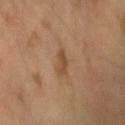follow-up: imaged on a skin check; not biopsied
image: 15 mm crop, total-body photography
patient: male, aged 63 to 67
diameter: ≈3 mm
lighting: cross-polarized illumination
automated lesion analysis: a footprint of about 3 mm², an eccentricity of roughly 0.85, and a shape-asymmetry score of about 0.35 (0 = symmetric)